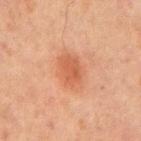| key | value |
|---|---|
| notes | catalogued during a skin exam; not biopsied |
| patient | male, about 65 years old |
| acquisition | ~15 mm crop, total-body skin-cancer survey |
| site | the front of the torso |